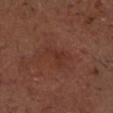{"biopsy_status": "not biopsied; imaged during a skin examination", "patient": {"sex": "male", "age_approx": 75}, "site": "head or neck", "image": {"source": "total-body photography crop", "field_of_view_mm": 15}}